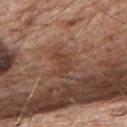Captured during whole-body skin photography for melanoma surveillance; the lesion was not biopsied. A male patient, aged 78–82. Located on the upper back. A close-up tile cropped from a whole-body skin photograph, about 15 mm across. The tile uses white-light illumination.